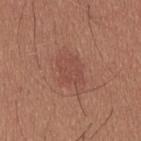workup — no biopsy performed (imaged during a skin exam)
image — ~15 mm crop, total-body skin-cancer survey
illumination — white-light illumination
TBP lesion metrics — a mean CIELAB color near L≈48 a*≈23 b*≈27 and about 6 CIELAB-L* units darker than the surrounding skin
size — about 5 mm
subject — male, aged 23 to 27
location — the upper back